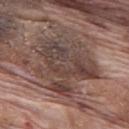workup: catalogued during a skin exam; not biopsied | illumination: white-light illumination | subject: male, aged 68–72 | body site: the mid back | TBP lesion metrics: a footprint of about 36 mm², a shape eccentricity near 0.25, and two-axis asymmetry of about 0.35; a mean CIELAB color near L≈42 a*≈16 b*≈21, roughly 10 lightness units darker than nearby skin, and a normalized border contrast of about 8.5 | lesion size: ≈7.5 mm | imaging modality: ~15 mm crop, total-body skin-cancer survey.